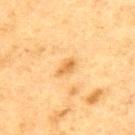| field | value |
|---|---|
| notes | total-body-photography surveillance lesion; no biopsy |
| subject | male, aged around 75 |
| image source | total-body-photography crop, ~15 mm field of view |
| lesion diameter | ≈2.5 mm |
| site | the mid back |